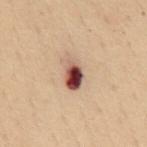Assessment:
The lesion was photographed on a routine skin check and not biopsied; there is no pathology result.
Acquisition and patient details:
From the mid back. About 4 mm across. Captured under cross-polarized illumination. A 15 mm close-up tile from a total-body photography series done for melanoma screening. A female subject, aged 48–52.